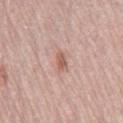Recorded during total-body skin imaging; not selected for excision or biopsy. A 15 mm crop from a total-body photograph taken for skin-cancer surveillance. An algorithmic analysis of the crop reported border irregularity of about 3.5 on a 0–10 scale and a peripheral color-asymmetry measure near 0.5. And it measured a lesion-detection confidence of about 100/100. The lesion's longest dimension is about 2.5 mm. The subject is a female aged approximately 65. Located on the left leg. This is a white-light tile.